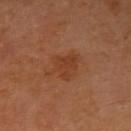Q: Was this lesion biopsied?
A: no biopsy performed (imaged during a skin exam)
Q: Automated lesion metrics?
A: an automated nevus-likeness rating near 15 out of 100
Q: What kind of image is this?
A: total-body-photography crop, ~15 mm field of view
Q: Where on the body is the lesion?
A: the right upper arm